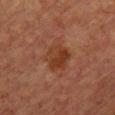Impression:
No biopsy was performed on this lesion — it was imaged during a full skin examination and was not determined to be concerning.